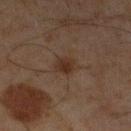Recorded during total-body skin imaging; not selected for excision or biopsy.
A 15 mm crop from a total-body photograph taken for skin-cancer surveillance.
Automated tile analysis of the lesion measured an area of roughly 4.5 mm² and a shape-asymmetry score of about 0.2 (0 = symmetric). And it measured a lesion-detection confidence of about 100/100.
From the left lower leg.
Captured under cross-polarized illumination.
A male subject in their mid-40s.